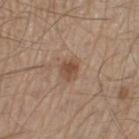No biopsy was performed on this lesion — it was imaged during a full skin examination and was not determined to be concerning. The lesion is on the left thigh. The tile uses white-light illumination. About 2.5 mm across. A male patient aged 58 to 62. A 15 mm close-up tile from a total-body photography series done for melanoma screening. An algorithmic analysis of the crop reported an eccentricity of roughly 0.5. The analysis additionally found border irregularity of about 1.5 on a 0–10 scale, a color-variation rating of about 2.5/10, and a peripheral color-asymmetry measure near 1.